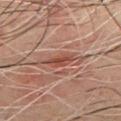No biopsy was performed on this lesion — it was imaged during a full skin examination and was not determined to be concerning.
The recorded lesion diameter is about 4 mm.
The subject is a male aged 58 to 62.
Automated tile analysis of the lesion measured an automated nevus-likeness rating near 0 out of 100 and a detector confidence of about 95 out of 100 that the crop contains a lesion.
Cropped from a whole-body photographic skin survey; the tile spans about 15 mm.
From the chest.
Captured under cross-polarized illumination.